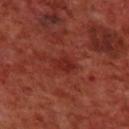Impression: Recorded during total-body skin imaging; not selected for excision or biopsy. Image and clinical context: The lesion is located on the upper back. The subject is a male in their 70s. This is a cross-polarized tile. Cropped from a whole-body photographic skin survey; the tile spans about 15 mm.